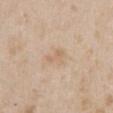Captured during whole-body skin photography for melanoma surveillance; the lesion was not biopsied. Cropped from a whole-body photographic skin survey; the tile spans about 15 mm. An algorithmic analysis of the crop reported a mean CIELAB color near L≈64 a*≈16 b*≈32, about 7 CIELAB-L* units darker than the surrounding skin, and a lesion-to-skin contrast of about 5 (normalized; higher = more distinct). The analysis additionally found a border-irregularity rating of about 4.5/10, a within-lesion color-variation index near 1/10, and a peripheral color-asymmetry measure near 0.5. The software also gave a classifier nevus-likeness of about 0/100 and a detector confidence of about 100 out of 100 that the crop contains a lesion. The subject is a female aged around 30. From the chest. This is a white-light tile.